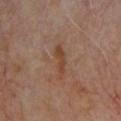Part of a total-body skin-imaging series; this lesion was reviewed on a skin check and was not flagged for biopsy.
A male patient aged 63–67.
This image is a 15 mm lesion crop taken from a total-body photograph.
Located on the chest.
Longest diameter approximately 4.5 mm.